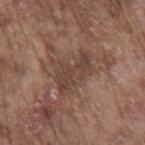The subject is a male aged 73 to 77. The total-body-photography lesion software estimated a lesion–skin lightness drop of about 8. It also reported a border-irregularity index near 8/10, a within-lesion color-variation index near 2/10, and a peripheral color-asymmetry measure near 0.5. The recorded lesion diameter is about 5 mm. Located on the mid back. A close-up tile cropped from a whole-body skin photograph, about 15 mm across. The tile uses white-light illumination.